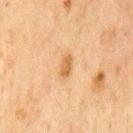Captured during whole-body skin photography for melanoma surveillance; the lesion was not biopsied. Cropped from a total-body skin-imaging series; the visible field is about 15 mm. The lesion is located on the front of the torso. About 3 mm across. The tile uses cross-polarized illumination. The patient is a male aged approximately 75.